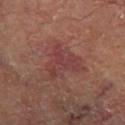{
  "biopsy_status": "not biopsied; imaged during a skin examination",
  "lighting": "cross-polarized",
  "image": {
    "source": "total-body photography crop",
    "field_of_view_mm": 15
  },
  "automated_metrics": {
    "shape_asymmetry": 0.55,
    "cielab_L": 39,
    "cielab_a": 25,
    "cielab_b": 21,
    "nevus_likeness_0_100": 0,
    "lesion_detection_confidence_0_100": 100
  },
  "site": "right thigh",
  "patient": {
    "sex": "male",
    "age_approx": 70
  }
}